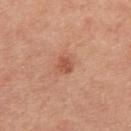  biopsy_status: not biopsied; imaged during a skin examination
  site: right upper arm
  lesion_size:
    long_diameter_mm_approx: 2.0
  automated_metrics:
    area_mm2_approx: 3.0
    eccentricity: 0.55
    cielab_L: 53
    cielab_a: 27
    cielab_b: 33
    vs_skin_darker_L: 10.0
    nevus_likeness_0_100: 65
    lesion_detection_confidence_0_100: 100
  patient:
    sex: female
    age_approx: 65
  lighting: white-light
  image:
    source: total-body photography crop
    field_of_view_mm: 15